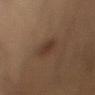Recorded during total-body skin imaging; not selected for excision or biopsy. The recorded lesion diameter is about 2.5 mm. An algorithmic analysis of the crop reported an area of roughly 4 mm², a shape eccentricity near 0.8, and a shape-asymmetry score of about 0.2 (0 = symmetric). It also reported border irregularity of about 2 on a 0–10 scale. Cropped from a total-body skin-imaging series; the visible field is about 15 mm. The tile uses cross-polarized illumination. A male subject aged approximately 30. From the left upper arm.